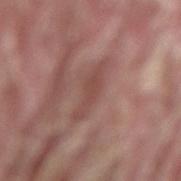The lesion was tiled from a total-body skin photograph and was not biopsied.
The lesion is on the lower back.
This image is a 15 mm lesion crop taken from a total-body photograph.
The tile uses white-light illumination.
An algorithmic analysis of the crop reported a footprint of about 6.5 mm², a shape eccentricity near 0.95, and a symmetry-axis asymmetry near 0.35. The analysis additionally found roughly 7 lightness units darker than nearby skin and a lesion-to-skin contrast of about 5.5 (normalized; higher = more distinct). It also reported a within-lesion color-variation index near 1/10 and peripheral color asymmetry of about 0.5.
A male subject aged 38 to 42.